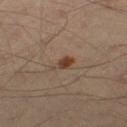Impression: Recorded during total-body skin imaging; not selected for excision or biopsy. Background: From the right thigh. The lesion-visualizer software estimated an area of roughly 3 mm², an eccentricity of roughly 0.9, and a shape-asymmetry score of about 0.3 (0 = symmetric). The analysis additionally found a lesion color around L≈35 a*≈16 b*≈25 in CIELAB, roughly 9 lightness units darker than nearby skin, and a lesion-to-skin contrast of about 9 (normalized; higher = more distinct). The software also gave border irregularity of about 3.5 on a 0–10 scale, internal color variation of about 2.5 on a 0–10 scale, and peripheral color asymmetry of about 0.5. A roughly 15 mm field-of-view crop from a total-body skin photograph. Approximately 3 mm at its widest. The subject is a male approximately 40 years of age. Captured under cross-polarized illumination.